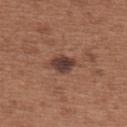  biopsy_status: not biopsied; imaged during a skin examination
  patient:
    sex: female
    age_approx: 65
  lesion_size:
    long_diameter_mm_approx: 3.0
  lighting: white-light
  site: upper back
  automated_metrics:
    area_mm2_approx: 5.0
    eccentricity: 0.7
    peripheral_color_asymmetry: 1.0
    nevus_likeness_0_100: 45
    lesion_detection_confidence_0_100: 100
  image:
    source: total-body photography crop
    field_of_view_mm: 15